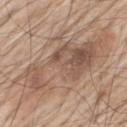Captured during whole-body skin photography for melanoma surveillance; the lesion was not biopsied. The patient is a male aged 68 to 72. From the back. A region of skin cropped from a whole-body photographic capture, roughly 15 mm wide.